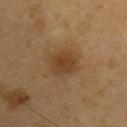The lesion was photographed on a routine skin check and not biopsied; there is no pathology result. The lesion-visualizer software estimated an area of roughly 8.5 mm², an eccentricity of roughly 0.6, and a symmetry-axis asymmetry near 0.2. It also reported a peripheral color-asymmetry measure near 1. A region of skin cropped from a whole-body photographic capture, roughly 15 mm wide. A male subject, approximately 60 years of age. The lesion is located on the left upper arm. Approximately 3.5 mm at its widest.